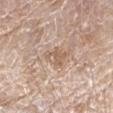Impression:
Captured during whole-body skin photography for melanoma surveillance; the lesion was not biopsied.
Clinical summary:
Located on the right forearm. A male subject aged around 80. Measured at roughly 3 mm in maximum diameter. A 15 mm close-up tile from a total-body photography series done for melanoma screening. Captured under white-light illumination.